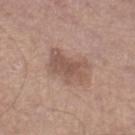notes=total-body-photography surveillance lesion; no biopsy